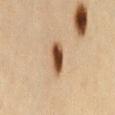biopsy status: total-body-photography surveillance lesion; no biopsy
automated metrics: an average lesion color of about L≈42 a*≈18 b*≈31 (CIELAB), a lesion–skin lightness drop of about 18, and a normalized lesion–skin contrast near 13.5
illumination: cross-polarized
lesion diameter: ~3.5 mm (longest diameter)
location: the lower back
imaging modality: ~15 mm tile from a whole-body skin photo
patient: female, aged 43–47